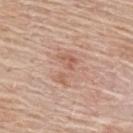The lesion was photographed on a routine skin check and not biopsied; there is no pathology result. A region of skin cropped from a whole-body photographic capture, roughly 15 mm wide. A female subject about 65 years old. On the upper back. Automated image analysis of the tile measured an area of roughly 9.5 mm², an eccentricity of roughly 0.8, and two-axis asymmetry of about 0.4. It also reported a nevus-likeness score of about 0/100 and lesion-presence confidence of about 100/100.